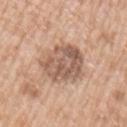Q: Was this lesion biopsied?
A: total-body-photography surveillance lesion; no biopsy
Q: What lighting was used for the tile?
A: white-light illumination
Q: Patient demographics?
A: male, about 50 years old
Q: What kind of image is this?
A: total-body-photography crop, ~15 mm field of view
Q: Lesion size?
A: about 6 mm
Q: Lesion location?
A: the left upper arm